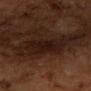biopsy status = catalogued during a skin exam; not biopsied | acquisition = 15 mm crop, total-body photography | subject = male, aged around 60 | site = the left forearm | illumination = cross-polarized | automated lesion analysis = an area of roughly 20 mm², a shape eccentricity near 0.9, and a symmetry-axis asymmetry near 0.35; a within-lesion color-variation index near 3.5/10 and radial color variation of about 1; a nevus-likeness score of about 0/100 and a lesion-detection confidence of about 80/100 | diameter = ≈7.5 mm.